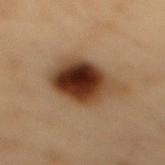biopsy status = catalogued during a skin exam; not biopsied.